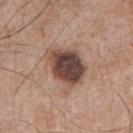A male patient in their mid-60s. Cropped from a total-body skin-imaging series; the visible field is about 15 mm. Captured under white-light illumination. Longest diameter approximately 5.5 mm. On the arm.